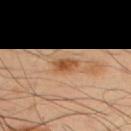The lesion was photographed on a routine skin check and not biopsied; there is no pathology result. The lesion is located on the back. This is a cross-polarized tile. A 15 mm crop from a total-body photograph taken for skin-cancer surveillance. The total-body-photography lesion software estimated a lesion area of about 5 mm² and a shape eccentricity near 0.85. The analysis additionally found a lesion color around L≈52 a*≈22 b*≈36 in CIELAB. The patient is a male aged approximately 50. Measured at roughly 3 mm in maximum diameter.